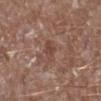Impression: Captured during whole-body skin photography for melanoma surveillance; the lesion was not biopsied. Image and clinical context: The tile uses white-light illumination. Automated image analysis of the tile measured an area of roughly 4.5 mm². It also reported an average lesion color of about L≈45 a*≈20 b*≈25 (CIELAB), about 7 CIELAB-L* units darker than the surrounding skin, and a lesion-to-skin contrast of about 5.5 (normalized; higher = more distinct). It also reported border irregularity of about 2.5 on a 0–10 scale, internal color variation of about 3 on a 0–10 scale, and peripheral color asymmetry of about 1. The analysis additionally found an automated nevus-likeness rating near 0 out of 100 and a lesion-detection confidence of about 100/100. Measured at roughly 3 mm in maximum diameter. On the right lower leg. The subject is a male aged 43–47. A lesion tile, about 15 mm wide, cut from a 3D total-body photograph.